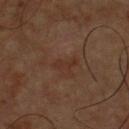Clinical impression:
The lesion was tiled from a total-body skin photograph and was not biopsied.
Acquisition and patient details:
The recorded lesion diameter is about 3 mm. From the chest. A close-up tile cropped from a whole-body skin photograph, about 15 mm across. Captured under cross-polarized illumination. A male subject aged approximately 60.